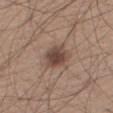This lesion was catalogued during total-body skin photography and was not selected for biopsy. The lesion is on the right thigh. The recorded lesion diameter is about 3.5 mm. The patient is a male in their 60s. Cropped from a total-body skin-imaging series; the visible field is about 15 mm. Imaged with white-light lighting.